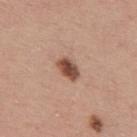follow-up: catalogued during a skin exam; not biopsied
subject: male, roughly 40 years of age
location: the upper back
image source: ~15 mm crop, total-body skin-cancer survey
lesion diameter: ≈3 mm
tile lighting: white-light